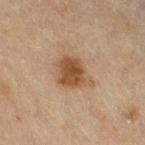Imaged during a routine full-body skin examination; the lesion was not biopsied and no histopathology is available. A 15 mm crop from a total-body photograph taken for skin-cancer surveillance. Located on the right thigh. The subject is a female aged 53–57. The tile uses cross-polarized illumination. The lesion's longest dimension is about 4.5 mm.